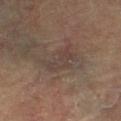| field | value |
|---|---|
| follow-up | no biopsy performed (imaged during a skin exam) |
| lesion diameter | ~4 mm (longest diameter) |
| site | the left lower leg |
| patient | female, roughly 80 years of age |
| image | ~15 mm crop, total-body skin-cancer survey |
| image-analysis metrics | a lesion color around L≈33 a*≈12 b*≈18 in CIELAB, a lesion–skin lightness drop of about 4, and a normalized border contrast of about 5 |
| tile lighting | cross-polarized illumination |